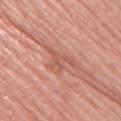biopsy_status: not biopsied; imaged during a skin examination
image:
  source: total-body photography crop
  field_of_view_mm: 15
site: upper back
automated_metrics:
  eccentricity: 0.8
  shape_asymmetry: 0.65
  border_irregularity_0_10: 8.5
  peripheral_color_asymmetry: 0.5
patient:
  sex: female
  age_approx: 60